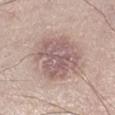Notes:
• notes · imaged on a skin check; not biopsied
• lesion diameter · about 6 mm
• tile lighting · white-light illumination
• site · the right lower leg
• subject · male, aged 43–47
• imaging modality · ~15 mm tile from a whole-body skin photo